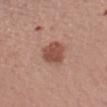{"biopsy_status": "not biopsied; imaged during a skin examination", "lesion_size": {"long_diameter_mm_approx": 4.0}, "image": {"source": "total-body photography crop", "field_of_view_mm": 15}, "site": "left forearm", "patient": {"sex": "male", "age_approx": 60}, "lighting": "white-light"}